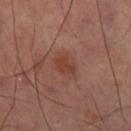Assessment: Part of a total-body skin-imaging series; this lesion was reviewed on a skin check and was not flagged for biopsy. Background: Imaged with cross-polarized lighting. A region of skin cropped from a whole-body photographic capture, roughly 15 mm wide. From the leg. Approximately 2.5 mm at its widest. Automated tile analysis of the lesion measured an average lesion color of about L≈41 a*≈25 b*≈29 (CIELAB), a lesion–skin lightness drop of about 7, and a lesion-to-skin contrast of about 6.5 (normalized; higher = more distinct). And it measured an automated nevus-likeness rating near 60 out of 100 and a lesion-detection confidence of about 100/100.